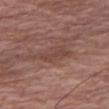A 15 mm close-up extracted from a 3D total-body photography capture. Automated tile analysis of the lesion measured roughly 7 lightness units darker than nearby skin and a lesion-to-skin contrast of about 5.5 (normalized; higher = more distinct). The tile uses white-light illumination. The patient is a male about 80 years old. Longest diameter approximately 4 mm. The lesion is on the right thigh.